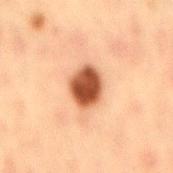Assessment: Captured during whole-body skin photography for melanoma surveillance; the lesion was not biopsied. Context: This is a cross-polarized tile. From the mid back. A close-up tile cropped from a whole-body skin photograph, about 15 mm across. A male subject, aged 48–52. Measured at roughly 4 mm in maximum diameter.